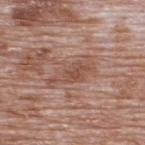This lesion was catalogued during total-body skin photography and was not selected for biopsy.
Captured under white-light illumination.
A roughly 15 mm field-of-view crop from a total-body skin photograph.
On the upper back.
A male patient aged 68–72.